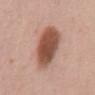| feature | finding |
|---|---|
| biopsy status | catalogued during a skin exam; not biopsied |
| image | ~15 mm tile from a whole-body skin photo |
| lesion diameter | ~6.5 mm (longest diameter) |
| body site | the mid back |
| lighting | white-light illumination |
| subject | female, in their 60s |